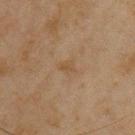Notes:
• notes · no biopsy performed (imaged during a skin exam)
• tile lighting · cross-polarized illumination
• site · the chest
• imaging modality · ~15 mm crop, total-body skin-cancer survey
• subject · male, approximately 45 years of age
• size · about 2.5 mm